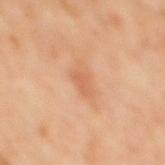| feature | finding |
|---|---|
| notes | catalogued during a skin exam; not biopsied |
| illumination | cross-polarized |
| patient | male, roughly 60 years of age |
| location | the mid back |
| imaging modality | total-body-photography crop, ~15 mm field of view |
| diameter | ~2.5 mm (longest diameter) |